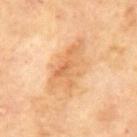Q: Is there a histopathology result?
A: catalogued during a skin exam; not biopsied
Q: Illumination type?
A: cross-polarized
Q: What are the patient's age and sex?
A: male, approximately 70 years of age
Q: What is the anatomic site?
A: the mid back
Q: What kind of image is this?
A: ~15 mm crop, total-body skin-cancer survey
Q: What did automated image analysis measure?
A: a mean CIELAB color near L≈67 a*≈22 b*≈41 and roughly 9 lightness units darker than nearby skin
Q: What is the lesion's diameter?
A: about 7 mm